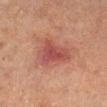notes: catalogued during a skin exam; not biopsied
anatomic site: the left lower leg
patient: male, about 65 years old
imaging modality: ~15 mm tile from a whole-body skin photo
illumination: cross-polarized illumination
TBP lesion metrics: roughly 8 lightness units darker than nearby skin and a normalized lesion–skin contrast near 7.5; border irregularity of about 4 on a 0–10 scale and a color-variation rating of about 3.5/10
lesion size: ≈4.5 mm